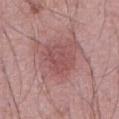The lesion was photographed on a routine skin check and not biopsied; there is no pathology result. A 15 mm close-up tile from a total-body photography series done for melanoma screening. The lesion is on the abdomen. A male patient, roughly 70 years of age. Measured at roughly 4 mm in maximum diameter. Captured under white-light illumination.